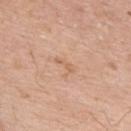{"biopsy_status": "not biopsied; imaged during a skin examination", "patient": {"sex": "male", "age_approx": 60}, "lighting": "white-light", "image": {"source": "total-body photography crop", "field_of_view_mm": 15}, "lesion_size": {"long_diameter_mm_approx": 2.5}, "site": "upper back"}